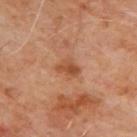Case summary:
– image — ~15 mm tile from a whole-body skin photo
– subject — male, aged 63 to 67
– lesion diameter — about 2.5 mm
– location — the upper back
– automated lesion analysis — a border-irregularity rating of about 3/10, a within-lesion color-variation index near 3/10, and a peripheral color-asymmetry measure near 1
– illumination — cross-polarized illumination